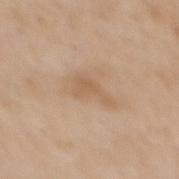follow-up — imaged on a skin check; not biopsied | image source — ~15 mm tile from a whole-body skin photo | subject — female, aged approximately 65 | site — the mid back.